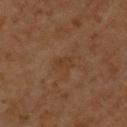This lesion was catalogued during total-body skin photography and was not selected for biopsy. A 15 mm close-up extracted from a 3D total-body photography capture. A male patient, roughly 60 years of age. About 3 mm across. The lesion is located on the chest. The tile uses cross-polarized illumination.